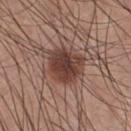Imaged during a routine full-body skin examination; the lesion was not biopsied and no histopathology is available.
The lesion-visualizer software estimated about 13 CIELAB-L* units darker than the surrounding skin and a normalized lesion–skin contrast near 10. And it measured a border-irregularity rating of about 2.5/10 and radial color variation of about 1.5. And it measured a lesion-detection confidence of about 100/100.
The recorded lesion diameter is about 5.5 mm.
Captured under white-light illumination.
A male subject aged approximately 25.
On the chest.
A close-up tile cropped from a whole-body skin photograph, about 15 mm across.